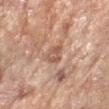Assessment:
Imaged during a routine full-body skin examination; the lesion was not biopsied and no histopathology is available.
Clinical summary:
Approximately 3 mm at its widest. The lesion is located on the arm. A female patient in their mid- to late 70s. A close-up tile cropped from a whole-body skin photograph, about 15 mm across.